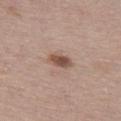Notes:
– site: the left thigh
– illumination: white-light illumination
– subject: female, aged approximately 50
– acquisition: ~15 mm crop, total-body skin-cancer survey